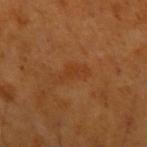Impression:
Part of a total-body skin-imaging series; this lesion was reviewed on a skin check and was not flagged for biopsy.
Background:
A male subject, aged approximately 50. The tile uses cross-polarized illumination. This image is a 15 mm lesion crop taken from a total-body photograph. The lesion is on the left forearm. Longest diameter approximately 3.5 mm.